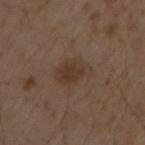| key | value |
|---|---|
| biopsy status | no biopsy performed (imaged during a skin exam) |
| size | about 4 mm |
| imaging modality | 15 mm crop, total-body photography |
| illumination | white-light illumination |
| automated lesion analysis | a footprint of about 9 mm², an outline eccentricity of about 0.75 (0 = round, 1 = elongated), and a shape-asymmetry score of about 0.25 (0 = symmetric); an automated nevus-likeness rating near 65 out of 100 |
| location | the left upper arm |
| subject | male, roughly 50 years of age |